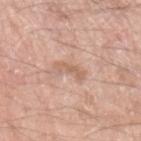notes — imaged on a skin check; not biopsied
patient — male, approximately 60 years of age
lighting — white-light
site — the arm
diameter — about 3.5 mm
imaging modality — 15 mm crop, total-body photography